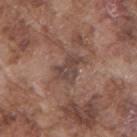No biopsy was performed on this lesion — it was imaged during a full skin examination and was not determined to be concerning.
About 4 mm across.
Captured under white-light illumination.
The lesion-visualizer software estimated a shape eccentricity near 0.75 and a shape-asymmetry score of about 0.4 (0 = symmetric). It also reported about 8 CIELAB-L* units darker than the surrounding skin and a normalized lesion–skin contrast near 7.
The subject is a male in their mid- to late 70s.
On the right upper arm.
This image is a 15 mm lesion crop taken from a total-body photograph.